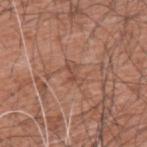Impression:
Imaged during a routine full-body skin examination; the lesion was not biopsied and no histopathology is available.
Acquisition and patient details:
A male subject, roughly 60 years of age. A 15 mm close-up extracted from a 3D total-body photography capture. Located on the upper back.